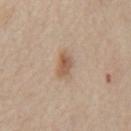biopsy status: imaged on a skin check; not biopsied | illumination: white-light | location: the abdomen | automated lesion analysis: an area of roughly 5 mm² and a symmetry-axis asymmetry near 0.3; a lesion color around L≈57 a*≈17 b*≈31 in CIELAB and a lesion–skin lightness drop of about 10; border irregularity of about 3 on a 0–10 scale and peripheral color asymmetry of about 1.5; a nevus-likeness score of about 80/100 | lesion diameter: about 3 mm | subject: male, aged 58 to 62 | image source: ~15 mm crop, total-body skin-cancer survey.